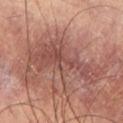Assessment: Captured during whole-body skin photography for melanoma surveillance; the lesion was not biopsied. Acquisition and patient details: On the left thigh. Automated image analysis of the tile measured an area of roughly 26 mm², an outline eccentricity of about 0.9 (0 = round, 1 = elongated), and a symmetry-axis asymmetry near 0.45. The analysis additionally found an average lesion color of about L≈49 a*≈23 b*≈24 (CIELAB), a lesion–skin lightness drop of about 10, and a normalized lesion–skin contrast near 7.5. A male patient, in their mid- to late 60s. The lesion's longest dimension is about 10 mm. A lesion tile, about 15 mm wide, cut from a 3D total-body photograph.